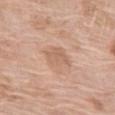workup: imaged on a skin check; not biopsied | size: ~3 mm (longest diameter) | tile lighting: white-light | anatomic site: the left thigh | acquisition: ~15 mm tile from a whole-body skin photo | automated lesion analysis: an outline eccentricity of about 0.5 (0 = round, 1 = elongated) and a shape-asymmetry score of about 0.2 (0 = symmetric); border irregularity of about 2 on a 0–10 scale, a within-lesion color-variation index near 2/10, and a peripheral color-asymmetry measure near 0.5 | subject: female, aged 73 to 77.